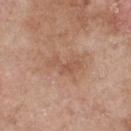notes = catalogued during a skin exam; not biopsied | acquisition = 15 mm crop, total-body photography | subject = male, aged around 55 | lighting = white-light illumination | anatomic site = the back | lesion diameter = ≈4 mm.